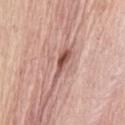Context:
A roughly 15 mm field-of-view crop from a total-body skin photograph. About 4.5 mm across. The subject is a female in their mid- to late 60s. On the abdomen.